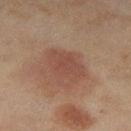Findings:
• workup · imaged on a skin check; not biopsied
• tile lighting · cross-polarized
• diameter · ≈5 mm
• location · the left thigh
• TBP lesion metrics · an area of roughly 11 mm², a shape eccentricity near 0.8, and a shape-asymmetry score of about 0.2 (0 = symmetric)
• acquisition · 15 mm crop, total-body photography
• subject · female, aged 58 to 62